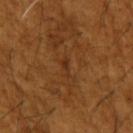Recorded during total-body skin imaging; not selected for excision or biopsy. A close-up tile cropped from a whole-body skin photograph, about 15 mm across. The lesion is located on the left upper arm. A male subject, approximately 65 years of age.